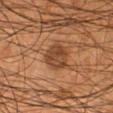imaging modality: 15 mm crop, total-body photography
patient: male, in their mid- to late 50s
automated lesion analysis: an eccentricity of roughly 0.55; a border-irregularity rating of about 2.5/10, internal color variation of about 3.5 on a 0–10 scale, and a peripheral color-asymmetry measure near 1
location: the right lower leg
illumination: cross-polarized